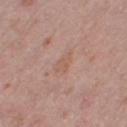Assessment: Captured during whole-body skin photography for melanoma surveillance; the lesion was not biopsied. Image and clinical context: A lesion tile, about 15 mm wide, cut from a 3D total-body photograph. The subject is a female roughly 40 years of age. This is a white-light tile. The recorded lesion diameter is about 2.5 mm. The lesion is located on the right thigh.